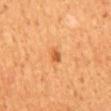Impression: No biopsy was performed on this lesion — it was imaged during a full skin examination and was not determined to be concerning. Context: The lesion's longest dimension is about 2 mm. On the back. An algorithmic analysis of the crop reported an eccentricity of roughly 0.8 and a shape-asymmetry score of about 0.3 (0 = symmetric). The software also gave a border-irregularity rating of about 2.5/10, a color-variation rating of about 1/10, and a peripheral color-asymmetry measure near 0.5. And it measured an automated nevus-likeness rating near 70 out of 100 and a lesion-detection confidence of about 100/100. A 15 mm crop from a total-body photograph taken for skin-cancer surveillance. The tile uses cross-polarized illumination. A male patient, in their mid-40s.